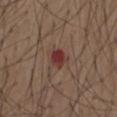Q: Was a biopsy performed?
A: total-body-photography surveillance lesion; no biopsy
Q: Where on the body is the lesion?
A: the abdomen
Q: What is the imaging modality?
A: ~15 mm crop, total-body skin-cancer survey
Q: Who is the patient?
A: male, aged approximately 75